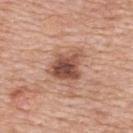The lesion was tiled from a total-body skin photograph and was not biopsied.
Cropped from a whole-body photographic skin survey; the tile spans about 15 mm.
The total-body-photography lesion software estimated an area of roughly 11 mm², an outline eccentricity of about 0.6 (0 = round, 1 = elongated), and two-axis asymmetry of about 0.25.
The lesion is located on the upper back.
Approximately 4.5 mm at its widest.
A female patient, approximately 60 years of age.
This is a white-light tile.